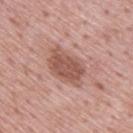Captured during whole-body skin photography for melanoma surveillance; the lesion was not biopsied. Imaged with white-light lighting. About 4.5 mm across. A 15 mm close-up extracted from a 3D total-body photography capture. From the upper back. A male subject, approximately 75 years of age.